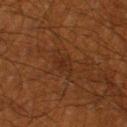This lesion was catalogued during total-body skin photography and was not selected for biopsy. From the leg. A region of skin cropped from a whole-body photographic capture, roughly 15 mm wide. The subject is a male aged approximately 60. Automated tile analysis of the lesion measured a classifier nevus-likeness of about 0/100 and a detector confidence of about 85 out of 100 that the crop contains a lesion. Approximately 2.5 mm at its widest. This is a cross-polarized tile.